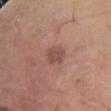<record>
  <biopsy_status>not biopsied; imaged during a skin examination</biopsy_status>
  <lighting>white-light</lighting>
  <automated_metrics>
    <area_mm2_approx>4.5</area_mm2_approx>
    <eccentricity>0.7</eccentricity>
    <color_variation_0_10>1.5</color_variation_0_10>
    <peripheral_color_asymmetry>0.5</peripheral_color_asymmetry>
  </automated_metrics>
  <patient>
    <sex>male</sex>
    <age_approx>60</age_approx>
  </patient>
  <site>right forearm</site>
  <image>
    <source>total-body photography crop</source>
    <field_of_view_mm>15</field_of_view_mm>
  </image>
</record>